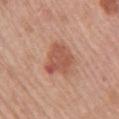Clinical impression: This lesion was catalogued during total-body skin photography and was not selected for biopsy. Context: Longest diameter approximately 4 mm. Automated image analysis of the tile measured a lesion area of about 10 mm², an eccentricity of roughly 0.65, and two-axis asymmetry of about 0.35. The software also gave an automated nevus-likeness rating near 95 out of 100 and a lesion-detection confidence of about 100/100. The lesion is on the right upper arm. This is a white-light tile. A female subject aged 63–67. A region of skin cropped from a whole-body photographic capture, roughly 15 mm wide.